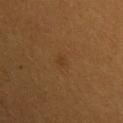Imaged during a routine full-body skin examination; the lesion was not biopsied and no histopathology is available.
The lesion's longest dimension is about 1.5 mm.
An algorithmic analysis of the crop reported a lesion area of about 1 mm², an eccentricity of roughly 0.75, and a shape-asymmetry score of about 0.6 (0 = symmetric). And it measured a lesion color around L≈33 a*≈18 b*≈32 in CIELAB and a lesion-to-skin contrast of about 4.5 (normalized; higher = more distinct).
Captured under cross-polarized illumination.
A female patient about 30 years old.
The lesion is located on the left upper arm.
A 15 mm close-up extracted from a 3D total-body photography capture.